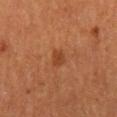automated_metrics:
  eccentricity: 0.7
  shape_asymmetry: 0.25
  cielab_L: 38
  cielab_a: 24
  cielab_b: 33
  vs_skin_contrast_norm: 6.5
  border_irregularity_0_10: 2.0
  color_variation_0_10: 1.5
  peripheral_color_asymmetry: 0.5
  nevus_likeness_0_100: 15
site: mid back
patient:
  sex: male
  age_approx: 65
image:
  source: total-body photography crop
  field_of_view_mm: 15
lighting: cross-polarized